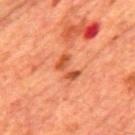biopsy_status: not biopsied; imaged during a skin examination